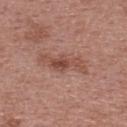The lesion was tiled from a total-body skin photograph and was not biopsied.
The tile uses white-light illumination.
The subject is a female aged approximately 50.
The lesion is located on the back.
A 15 mm close-up tile from a total-body photography series done for melanoma screening.